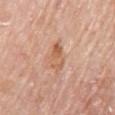Recorded during total-body skin imaging; not selected for excision or biopsy. The tile uses white-light illumination. Measured at roughly 3.5 mm in maximum diameter. Located on the right upper arm. The subject is a female in their mid-60s. An algorithmic analysis of the crop reported a lesion area of about 6 mm² and a shape-asymmetry score of about 0.25 (0 = symmetric). The software also gave a lesion color around L≈60 a*≈22 b*≈34 in CIELAB, a lesion–skin lightness drop of about 8, and a lesion-to-skin contrast of about 6.5 (normalized; higher = more distinct). The software also gave a lesion-detection confidence of about 100/100. Cropped from a whole-body photographic skin survey; the tile spans about 15 mm.